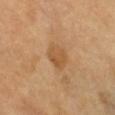Impression:
Recorded during total-body skin imaging; not selected for excision or biopsy.
Acquisition and patient details:
On the chest. A lesion tile, about 15 mm wide, cut from a 3D total-body photograph. A female subject roughly 65 years of age.